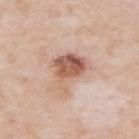Captured during whole-body skin photography for melanoma surveillance; the lesion was not biopsied.
Automated tile analysis of the lesion measured a lesion area of about 13 mm², an eccentricity of roughly 0.7, and a shape-asymmetry score of about 0.4 (0 = symmetric). The analysis additionally found roughly 13 lightness units darker than nearby skin and a normalized lesion–skin contrast near 8.5. The analysis additionally found an automated nevus-likeness rating near 40 out of 100 and a detector confidence of about 100 out of 100 that the crop contains a lesion.
Cropped from a whole-body photographic skin survey; the tile spans about 15 mm.
The lesion's longest dimension is about 5 mm.
A male patient aged approximately 50.
From the back.